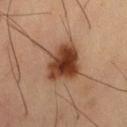Q: Is there a histopathology result?
A: catalogued during a skin exam; not biopsied
Q: Who is the patient?
A: male, aged 58–62
Q: What is the anatomic site?
A: the right thigh
Q: What is the imaging modality?
A: ~15 mm crop, total-body skin-cancer survey
Q: Lesion size?
A: ~5 mm (longest diameter)
Q: Illumination type?
A: cross-polarized